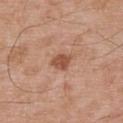Findings:
- workup: catalogued during a skin exam; not biopsied
- subject: male, aged around 55
- lighting: white-light illumination
- body site: the back
- image: ~15 mm tile from a whole-body skin photo
- TBP lesion metrics: a border-irregularity index near 2.5/10, internal color variation of about 2 on a 0–10 scale, and peripheral color asymmetry of about 0.5; a classifier nevus-likeness of about 60/100